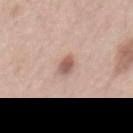Clinical impression: The lesion was tiled from a total-body skin photograph and was not biopsied. Acquisition and patient details: The patient is a male aged approximately 50. A 15 mm close-up extracted from a 3D total-body photography capture. From the abdomen.